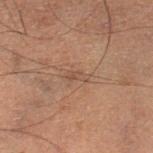Notes:
• workup · imaged on a skin check; not biopsied
• acquisition · ~15 mm tile from a whole-body skin photo
• location · the left lower leg
• tile lighting · cross-polarized illumination
• automated metrics · roughly 5 lightness units darker than nearby skin and a normalized lesion–skin contrast near 5; border irregularity of about 3.5 on a 0–10 scale, internal color variation of about 0 on a 0–10 scale, and a peripheral color-asymmetry measure near 0
• lesion size · ~2.5 mm (longest diameter)
• subject · male, about 60 years old